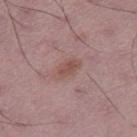notes=total-body-photography surveillance lesion; no biopsy
body site=the right thigh
patient=male, aged approximately 50
imaging modality=15 mm crop, total-body photography
size=about 2.5 mm
lighting=white-light illumination